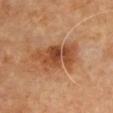Assessment:
Recorded during total-body skin imaging; not selected for excision or biopsy.
Image and clinical context:
An algorithmic analysis of the crop reported a mean CIELAB color near L≈46 a*≈24 b*≈35, roughly 11 lightness units darker than nearby skin, and a lesion-to-skin contrast of about 8.5 (normalized; higher = more distinct). The software also gave a border-irregularity rating of about 4.5/10 and a within-lesion color-variation index near 5/10. It also reported a nevus-likeness score of about 40/100 and lesion-presence confidence of about 100/100. The patient is a male aged approximately 60. Cropped from a total-body skin-imaging series; the visible field is about 15 mm. About 6 mm across. The lesion is located on the upper back.